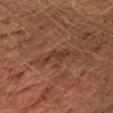The lesion was tiled from a total-body skin photograph and was not biopsied. A male subject about 75 years old. This image is a 15 mm lesion crop taken from a total-body photograph. An algorithmic analysis of the crop reported a footprint of about 4 mm², a shape eccentricity near 0.95, and a shape-asymmetry score of about 0.5 (0 = symmetric). The analysis additionally found a mean CIELAB color near L≈29 a*≈20 b*≈22, roughly 6 lightness units darker than nearby skin, and a normalized lesion–skin contrast near 6. The analysis additionally found a classifier nevus-likeness of about 0/100 and a lesion-detection confidence of about 80/100. Measured at roughly 3.5 mm in maximum diameter. Located on the left upper arm.